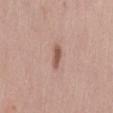Impression: No biopsy was performed on this lesion — it was imaged during a full skin examination and was not determined to be concerning. Context: The lesion is located on the lower back. Longest diameter approximately 3 mm. A female subject, approximately 40 years of age. A 15 mm close-up extracted from a 3D total-body photography capture.